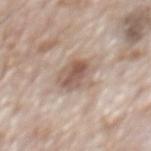Imaged with white-light lighting. A male patient aged 68 to 72. Longest diameter approximately 4 mm. A 15 mm close-up extracted from a 3D total-body photography capture. From the mid back.